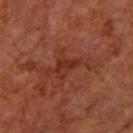Q: Was a biopsy performed?
A: catalogued during a skin exam; not biopsied
Q: Patient demographics?
A: female, aged around 65
Q: How large is the lesion?
A: about 3 mm
Q: What is the anatomic site?
A: the left forearm
Q: How was this image acquired?
A: 15 mm crop, total-body photography
Q: What did automated image analysis measure?
A: a classifier nevus-likeness of about 0/100